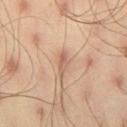The lesion was tiled from a total-body skin photograph and was not biopsied.
Cropped from a whole-body photographic skin survey; the tile spans about 15 mm.
A male subject aged 43–47.
The lesion-visualizer software estimated about 9 CIELAB-L* units darker than the surrounding skin and a normalized lesion–skin contrast near 6. The analysis additionally found border irregularity of about 3 on a 0–10 scale and radial color variation of about 0.5.
Imaged with cross-polarized lighting.
The lesion is located on the right thigh.
The lesion's longest dimension is about 3 mm.